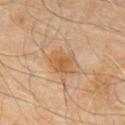Background: The patient is a male aged 58 to 62. On the chest. The total-body-photography lesion software estimated an area of roughly 7 mm², a shape eccentricity near 0.65, and a shape-asymmetry score of about 0.25 (0 = symmetric). The analysis additionally found an average lesion color of about L≈55 a*≈20 b*≈37 (CIELAB) and about 8 CIELAB-L* units darker than the surrounding skin. And it measured a border-irregularity index near 2.5/10, a within-lesion color-variation index near 3/10, and radial color variation of about 1. And it measured a detector confidence of about 100 out of 100 that the crop contains a lesion. Cropped from a total-body skin-imaging series; the visible field is about 15 mm. Longest diameter approximately 3.5 mm.